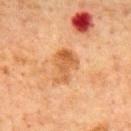Clinical impression: Recorded during total-body skin imaging; not selected for excision or biopsy. Acquisition and patient details: The lesion's longest dimension is about 4 mm. Cropped from a total-body skin-imaging series; the visible field is about 15 mm. A male patient aged approximately 65. Automated image analysis of the tile measured a shape-asymmetry score of about 0.4 (0 = symmetric). The software also gave a lesion color around L≈52 a*≈23 b*≈38 in CIELAB, about 9 CIELAB-L* units darker than the surrounding skin, and a normalized lesion–skin contrast near 7.5. The analysis additionally found internal color variation of about 4 on a 0–10 scale and peripheral color asymmetry of about 1.5. And it measured a nevus-likeness score of about 30/100 and a detector confidence of about 100 out of 100 that the crop contains a lesion. The lesion is located on the mid back.